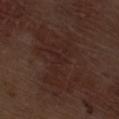Case summary:
- follow-up · total-body-photography surveillance lesion; no biopsy
- anatomic site · the right thigh
- patient · male, aged 68 to 72
- imaging modality · ~15 mm tile from a whole-body skin photo
- automated metrics · border irregularity of about 9 on a 0–10 scale and a color-variation rating of about 3/10; an automated nevus-likeness rating near 0 out of 100 and lesion-presence confidence of about 95/100